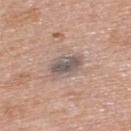Assessment:
No biopsy was performed on this lesion — it was imaged during a full skin examination and was not determined to be concerning.
Clinical summary:
From the upper back. A lesion tile, about 15 mm wide, cut from a 3D total-body photograph. Imaged with white-light lighting. Measured at roughly 4 mm in maximum diameter. The patient is a male aged around 70.